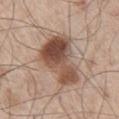Impression:
No biopsy was performed on this lesion — it was imaged during a full skin examination and was not determined to be concerning.
Acquisition and patient details:
About 7 mm across. The lesion is on the right thigh. The patient is a male roughly 60 years of age. A 15 mm close-up extracted from a 3D total-body photography capture.